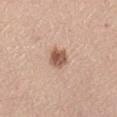biopsy status — no biopsy performed (imaged during a skin exam) | subject — female, aged 58 to 62 | image — ~15 mm tile from a whole-body skin photo | body site — the right lower leg.